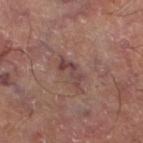Captured during whole-body skin photography for melanoma surveillance; the lesion was not biopsied.
The tile uses cross-polarized illumination.
The lesion is on the left thigh.
The lesion-visualizer software estimated a shape eccentricity near 0.9 and a symmetry-axis asymmetry near 0.5. The analysis additionally found a lesion–skin lightness drop of about 9 and a normalized border contrast of about 7.5. It also reported border irregularity of about 7 on a 0–10 scale, internal color variation of about 0 on a 0–10 scale, and peripheral color asymmetry of about 0. The software also gave a nevus-likeness score of about 0/100 and a detector confidence of about 100 out of 100 that the crop contains a lesion.
The recorded lesion diameter is about 3.5 mm.
A lesion tile, about 15 mm wide, cut from a 3D total-body photograph.